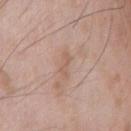Assessment:
This lesion was catalogued during total-body skin photography and was not selected for biopsy.
Acquisition and patient details:
On the chest. Automated image analysis of the tile measured an area of roughly 3 mm², an outline eccentricity of about 0.9 (0 = round, 1 = elongated), and a symmetry-axis asymmetry near 0.5. The software also gave a lesion color around L≈59 a*≈19 b*≈28 in CIELAB, about 7 CIELAB-L* units darker than the surrounding skin, and a normalized border contrast of about 5. And it measured a border-irregularity rating of about 5.5/10, a within-lesion color-variation index near 0/10, and peripheral color asymmetry of about 0. A region of skin cropped from a whole-body photographic capture, roughly 15 mm wide. Approximately 3 mm at its widest. A male patient aged 63 to 67. Captured under white-light illumination.